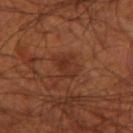<lesion>
<biopsy_status>not biopsied; imaged during a skin examination</biopsy_status>
<patient>
  <sex>male</sex>
  <age_approx>70</age_approx>
</patient>
<site>left thigh</site>
<image>
  <source>total-body photography crop</source>
  <field_of_view_mm>15</field_of_view_mm>
</image>
<automated_metrics>
  <vs_skin_contrast_norm>6.0</vs_skin_contrast_norm>
  <border_irregularity_0_10>3.0</border_irregularity_0_10>
  <color_variation_0_10>3.0</color_variation_0_10>
  <peripheral_color_asymmetry>1.0</peripheral_color_asymmetry>
  <nevus_likeness_0_100>5</nevus_likeness_0_100>
</automated_metrics>
<lesion_size>
  <long_diameter_mm_approx>3.0</long_diameter_mm_approx>
</lesion_size>
<lighting>cross-polarized</lighting>
</lesion>